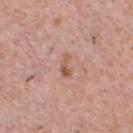{
  "biopsy_status": "not biopsied; imaged during a skin examination",
  "image": {
    "source": "total-body photography crop",
    "field_of_view_mm": 15
  },
  "site": "chest",
  "automated_metrics": {
    "cielab_L": 57,
    "cielab_a": 22,
    "cielab_b": 29,
    "vs_skin_darker_L": 9.0,
    "border_irregularity_0_10": 4.0,
    "color_variation_0_10": 1.0,
    "peripheral_color_asymmetry": 0.5
  },
  "lesion_size": {
    "long_diameter_mm_approx": 2.5
  },
  "lighting": "white-light",
  "patient": {
    "sex": "male",
    "age_approx": 40
  }
}